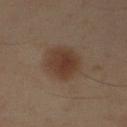- follow-up · catalogued during a skin exam; not biopsied
- TBP lesion metrics · a color-variation rating of about 3/10 and radial color variation of about 1; a classifier nevus-likeness of about 100/100
- tile lighting · cross-polarized
- subject · male, aged approximately 55
- site · the right upper arm
- acquisition · ~15 mm tile from a whole-body skin photo